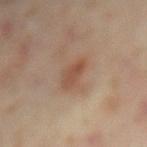Q: Was this lesion biopsied?
A: catalogued during a skin exam; not biopsied
Q: Who is the patient?
A: female, aged approximately 50
Q: Automated lesion metrics?
A: a footprint of about 6.5 mm² and two-axis asymmetry of about 0.25; roughly 8 lightness units darker than nearby skin and a normalized lesion–skin contrast near 6.5; an automated nevus-likeness rating near 60 out of 100
Q: How was this image acquired?
A: ~15 mm crop, total-body skin-cancer survey
Q: How large is the lesion?
A: about 3.5 mm
Q: What is the anatomic site?
A: the leg